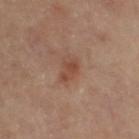Clinical impression: Imaged during a routine full-body skin examination; the lesion was not biopsied and no histopathology is available. Background: Located on the right upper arm. A region of skin cropped from a whole-body photographic capture, roughly 15 mm wide. The patient is a female approximately 65 years of age.